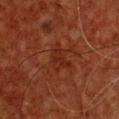biopsy_status: not biopsied; imaged during a skin examination
site: chest
patient:
  sex: male
  age_approx: 60
image:
  source: total-body photography crop
  field_of_view_mm: 15
lesion_size:
  long_diameter_mm_approx: 3.0